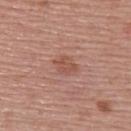Part of a total-body skin-imaging series; this lesion was reviewed on a skin check and was not flagged for biopsy. The lesion is on the upper back. About 2.5 mm across. This image is a 15 mm lesion crop taken from a total-body photograph. A female subject, in their mid- to late 50s. The tile uses white-light illumination.